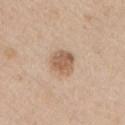The lesion was photographed on a routine skin check and not biopsied; there is no pathology result.
Imaged with white-light lighting.
From the left upper arm.
About 3.5 mm across.
A 15 mm crop from a total-body photograph taken for skin-cancer surveillance.
The patient is a male aged 58 to 62.